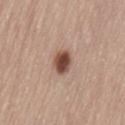notes: catalogued during a skin exam; not biopsied | location: the lower back | lesion diameter: ≈3 mm | lighting: white-light | image: ~15 mm crop, total-body skin-cancer survey | patient: female, aged around 75 | image-analysis metrics: an area of roughly 5.5 mm², a shape eccentricity near 0.6, and two-axis asymmetry of about 0.25; border irregularity of about 2 on a 0–10 scale and a within-lesion color-variation index near 4/10.